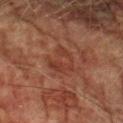Q: Was a biopsy performed?
A: total-body-photography surveillance lesion; no biopsy
Q: What kind of image is this?
A: ~15 mm tile from a whole-body skin photo
Q: What did automated image analysis measure?
A: a footprint of about 6.5 mm², an outline eccentricity of about 0.65 (0 = round, 1 = elongated), and a shape-asymmetry score of about 0.6 (0 = symmetric); roughly 5 lightness units darker than nearby skin and a normalized lesion–skin contrast near 5; border irregularity of about 9.5 on a 0–10 scale, a color-variation rating of about 2/10, and peripheral color asymmetry of about 0.5; a detector confidence of about 100 out of 100 that the crop contains a lesion
Q: Patient demographics?
A: male, roughly 75 years of age
Q: What is the anatomic site?
A: the left forearm
Q: What is the lesion's diameter?
A: about 4 mm